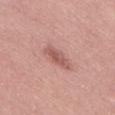Q: Is there a histopathology result?
A: catalogued during a skin exam; not biopsied
Q: What are the patient's age and sex?
A: male, aged around 50
Q: Lesion size?
A: ~3.5 mm (longest diameter)
Q: What is the imaging modality?
A: total-body-photography crop, ~15 mm field of view
Q: How was the tile lit?
A: white-light illumination
Q: Where on the body is the lesion?
A: the lower back